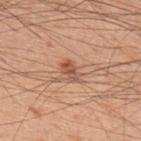The subject is a male in their 60s. A 15 mm crop from a total-body photograph taken for skin-cancer surveillance. From the upper back.